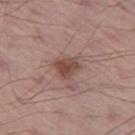No biopsy was performed on this lesion — it was imaged during a full skin examination and was not determined to be concerning.